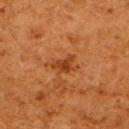Captured during whole-body skin photography for melanoma surveillance; the lesion was not biopsied.
The subject is a female aged 48 to 52.
The lesion is located on the upper back.
Longest diameter approximately 3 mm.
Cropped from a whole-body photographic skin survey; the tile spans about 15 mm.
The lesion-visualizer software estimated a footprint of about 4 mm² and a shape-asymmetry score of about 0.55 (0 = symmetric). The software also gave lesion-presence confidence of about 100/100.
This is a cross-polarized tile.